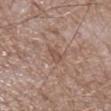Impression: Imaged during a routine full-body skin examination; the lesion was not biopsied and no histopathology is available. Image and clinical context: A 15 mm crop from a total-body photograph taken for skin-cancer surveillance. A male patient, aged approximately 65. The lesion is located on the right lower leg. An algorithmic analysis of the crop reported a border-irregularity index near 3/10 and a within-lesion color-variation index near 0.5/10. About 2.5 mm across. Captured under white-light illumination.